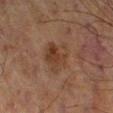No biopsy was performed on this lesion — it was imaged during a full skin examination and was not determined to be concerning. Longest diameter approximately 4.5 mm. A 15 mm close-up tile from a total-body photography series done for melanoma screening. Imaged with cross-polarized lighting. From the right lower leg. A male subject, aged around 70. Automated tile analysis of the lesion measured a border-irregularity rating of about 2.5/10, internal color variation of about 4.5 on a 0–10 scale, and peripheral color asymmetry of about 1.5. The analysis additionally found a nevus-likeness score of about 5/100 and lesion-presence confidence of about 100/100.